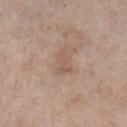The lesion is on the left lower leg. Imaged with white-light lighting. A lesion tile, about 15 mm wide, cut from a 3D total-body photograph. A female patient roughly 75 years of age.